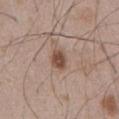Assessment: This lesion was catalogued during total-body skin photography and was not selected for biopsy. Clinical summary: Cropped from a total-body skin-imaging series; the visible field is about 15 mm. Located on the abdomen. A male patient, aged around 50.